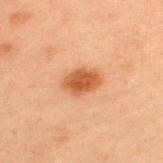Context:
From the upper back. A male patient in their mid- to late 50s. Cropped from a whole-body photographic skin survey; the tile spans about 15 mm.